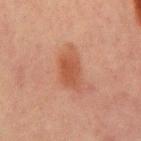Impression: The lesion was photographed on a routine skin check and not biopsied; there is no pathology result. Acquisition and patient details: An algorithmic analysis of the crop reported an average lesion color of about L≈42 a*≈22 b*≈28 (CIELAB), a lesion–skin lightness drop of about 7, and a normalized border contrast of about 6.5. The analysis additionally found border irregularity of about 3 on a 0–10 scale, a within-lesion color-variation index near 2.5/10, and radial color variation of about 0.5. This image is a 15 mm lesion crop taken from a total-body photograph. The recorded lesion diameter is about 5.5 mm. A male subject about 65 years old. From the abdomen.